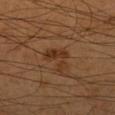Clinical impression:
Part of a total-body skin-imaging series; this lesion was reviewed on a skin check and was not flagged for biopsy.
Acquisition and patient details:
About 3.5 mm across. A male subject, aged around 60. Captured under cross-polarized illumination. The lesion is located on the left forearm. Automated tile analysis of the lesion measured a footprint of about 6 mm², a shape eccentricity near 0.65, and a symmetry-axis asymmetry near 0.55. A close-up tile cropped from a whole-body skin photograph, about 15 mm across.